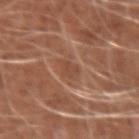| key | value |
|---|---|
| follow-up | total-body-photography surveillance lesion; no biopsy |
| image-analysis metrics | border irregularity of about 3 on a 0–10 scale, a color-variation rating of about 1.5/10, and radial color variation of about 0.5; a nevus-likeness score of about 0/100 and a detector confidence of about 65 out of 100 that the crop contains a lesion |
| subject | male, in their mid- to late 40s |
| image | total-body-photography crop, ~15 mm field of view |
| location | the arm |
| lesion diameter | ≈3 mm |